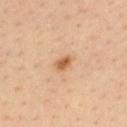Part of a total-body skin-imaging series; this lesion was reviewed on a skin check and was not flagged for biopsy.
A roughly 15 mm field-of-view crop from a total-body skin photograph.
From the upper back.
The lesion's longest dimension is about 2.5 mm.
Imaged with cross-polarized lighting.
An algorithmic analysis of the crop reported a footprint of about 3.5 mm² and a shape-asymmetry score of about 0.3 (0 = symmetric). The software also gave a classifier nevus-likeness of about 95/100.
The patient is a male roughly 35 years of age.